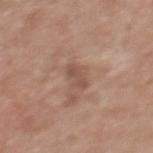• workup · imaged on a skin check; not biopsied
• subject · female, approximately 35 years of age
• acquisition · ~15 mm crop, total-body skin-cancer survey
• location · the upper back